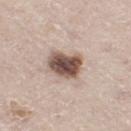Q: Was this lesion biopsied?
A: no biopsy performed (imaged during a skin exam)
Q: What lighting was used for the tile?
A: white-light illumination
Q: What is the anatomic site?
A: the right thigh
Q: What is the imaging modality?
A: 15 mm crop, total-body photography
Q: Patient demographics?
A: male, aged around 65
Q: What is the lesion's diameter?
A: ≈4 mm